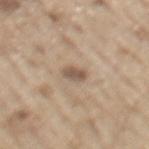Part of a total-body skin-imaging series; this lesion was reviewed on a skin check and was not flagged for biopsy. Measured at roughly 2.5 mm in maximum diameter. Cropped from a total-body skin-imaging series; the visible field is about 15 mm. Located on the mid back. Automated tile analysis of the lesion measured a footprint of about 3.5 mm² and a shape-asymmetry score of about 0.2 (0 = symmetric). And it measured a border-irregularity rating of about 2/10 and peripheral color asymmetry of about 0.5. A male patient, aged 68–72. Captured under white-light illumination.